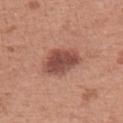Imaged during a routine full-body skin examination; the lesion was not biopsied and no histopathology is available. From the left upper arm. A lesion tile, about 15 mm wide, cut from a 3D total-body photograph. The lesion-visualizer software estimated an average lesion color of about L≈48 a*≈24 b*≈27 (CIELAB), a lesion–skin lightness drop of about 13, and a normalized border contrast of about 9. It also reported border irregularity of about 2 on a 0–10 scale, a within-lesion color-variation index near 3.5/10, and a peripheral color-asymmetry measure near 1. The lesion's longest dimension is about 4.5 mm. Imaged with white-light lighting. The subject is a female in their 60s.